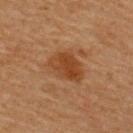<tbp_lesion>
  <patient>
    <sex>male</sex>
    <age_approx>65</age_approx>
  </patient>
  <image>
    <source>total-body photography crop</source>
    <field_of_view_mm>15</field_of_view_mm>
  </image>
  <site>upper back</site>
  <lesion_size>
    <long_diameter_mm_approx>4.0</long_diameter_mm_approx>
  </lesion_size>
</tbp_lesion>